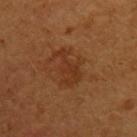Notes:
- biopsy status — catalogued during a skin exam; not biopsied
- tile lighting — cross-polarized
- diameter — about 4.5 mm
- automated lesion analysis — an average lesion color of about L≈31 a*≈21 b*≈31 (CIELAB), roughly 6 lightness units darker than nearby skin, and a normalized lesion–skin contrast near 6; a classifier nevus-likeness of about 5/100 and a detector confidence of about 100 out of 100 that the crop contains a lesion
- subject — male, aged approximately 55
- anatomic site — the left upper arm
- acquisition — 15 mm crop, total-body photography